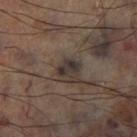Part of a total-body skin-imaging series; this lesion was reviewed on a skin check and was not flagged for biopsy. The lesion is on the right lower leg. The tile uses cross-polarized illumination. Measured at roughly 2.5 mm in maximum diameter. An algorithmic analysis of the crop reported an eccentricity of roughly 0.7 and two-axis asymmetry of about 0.2. It also reported roughly 10 lightness units darker than nearby skin and a normalized lesion–skin contrast near 11. And it measured an automated nevus-likeness rating near 0 out of 100 and lesion-presence confidence of about 50/100. This image is a 15 mm lesion crop taken from a total-body photograph.